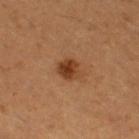Assessment:
Recorded during total-body skin imaging; not selected for excision or biopsy.
Background:
The patient is a female in their mid- to late 50s. A lesion tile, about 15 mm wide, cut from a 3D total-body photograph. An algorithmic analysis of the crop reported an average lesion color of about L≈41 a*≈24 b*≈35 (CIELAB), a lesion–skin lightness drop of about 12, and a lesion-to-skin contrast of about 9.5 (normalized; higher = more distinct). The analysis additionally found a border-irregularity index near 1.5/10, a color-variation rating of about 4/10, and peripheral color asymmetry of about 1.5. Imaged with cross-polarized lighting. The lesion is located on the right thigh. The recorded lesion diameter is about 3 mm.